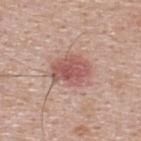The lesion was tiled from a total-body skin photograph and was not biopsied.